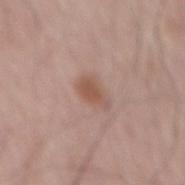Recorded during total-body skin imaging; not selected for excision or biopsy. Cropped from a whole-body photographic skin survey; the tile spans about 15 mm. The patient is a male aged around 65. The lesion is located on the mid back. The tile uses white-light illumination.